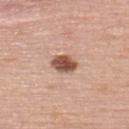workup = no biopsy performed (imaged during a skin exam) | patient = female, aged approximately 60 | site = the upper back | image = ~15 mm tile from a whole-body skin photo.